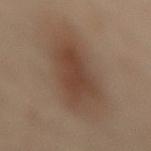This image is a 15 mm lesion crop taken from a total-body photograph. From the mid back. Imaged with cross-polarized lighting. The lesion's longest dimension is about 10 mm. A female subject in their mid-50s.